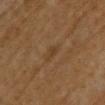notes: no biopsy performed (imaged during a skin exam); image source: total-body-photography crop, ~15 mm field of view; lesion diameter: ~3 mm (longest diameter); site: the left upper arm; illumination: cross-polarized; patient: female, aged around 55.